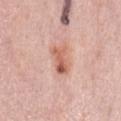biopsy status — no biopsy performed (imaged during a skin exam)